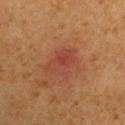Q: Was a biopsy performed?
A: no biopsy performed (imaged during a skin exam)
Q: Lesion size?
A: ≈4.5 mm
Q: Automated lesion metrics?
A: a footprint of about 8 mm² and an eccentricity of roughly 0.8; a mean CIELAB color near L≈38 a*≈24 b*≈28, about 6 CIELAB-L* units darker than the surrounding skin, and a lesion-to-skin contrast of about 5 (normalized; higher = more distinct); internal color variation of about 5 on a 0–10 scale and radial color variation of about 2
Q: Who is the patient?
A: male, aged around 65
Q: What kind of image is this?
A: ~15 mm crop, total-body skin-cancer survey
Q: Lesion location?
A: the left upper arm
Q: Illumination type?
A: cross-polarized illumination